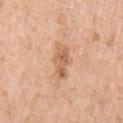image-analysis metrics: a lesion color around L≈62 a*≈22 b*≈35 in CIELAB, about 10 CIELAB-L* units darker than the surrounding skin, and a normalized border contrast of about 6.5; an automated nevus-likeness rating near 5 out of 100 and a lesion-detection confidence of about 100/100 | lighting: white-light | size: about 4 mm | image: total-body-photography crop, ~15 mm field of view | site: the left upper arm | patient: male, about 70 years old.